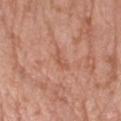notes = catalogued during a skin exam; not biopsied
image-analysis metrics = a border-irregularity index near 4.5/10, a within-lesion color-variation index near 0/10, and a peripheral color-asymmetry measure near 0; a detector confidence of about 100 out of 100 that the crop contains a lesion
diameter = ≈2.5 mm
image = total-body-photography crop, ~15 mm field of view
tile lighting = white-light illumination
subject = female, about 75 years old
site = the right forearm